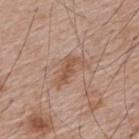Assessment: The lesion was tiled from a total-body skin photograph and was not biopsied. Context: A close-up tile cropped from a whole-body skin photograph, about 15 mm across. The lesion is on the upper back. The subject is a male in their mid- to late 60s. This is a white-light tile. About 4.5 mm across.